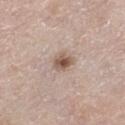The lesion was photographed on a routine skin check and not biopsied; there is no pathology result. The tile uses white-light illumination. The lesion is located on the right lower leg. The lesion-visualizer software estimated an area of roughly 4.5 mm² and a shape-asymmetry score of about 0.25 (0 = symmetric). The software also gave an average lesion color of about L≈55 a*≈16 b*≈26 (CIELAB). The software also gave a border-irregularity index near 2.5/10, internal color variation of about 5 on a 0–10 scale, and a peripheral color-asymmetry measure near 1.5. It also reported a detector confidence of about 100 out of 100 that the crop contains a lesion. A male subject aged 68 to 72. The lesion's longest dimension is about 2.5 mm. A close-up tile cropped from a whole-body skin photograph, about 15 mm across.